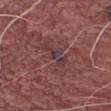notes = total-body-photography surveillance lesion; no biopsy | subject = male, about 80 years old | lesion diameter = ≈4 mm | image-analysis metrics = an area of roughly 5.5 mm², a shape eccentricity near 0.9, and two-axis asymmetry of about 0.5; a lesion color around L≈36 a*≈21 b*≈15 in CIELAB and a normalized lesion–skin contrast near 6.5; an automated nevus-likeness rating near 0 out of 100 and a detector confidence of about 95 out of 100 that the crop contains a lesion | anatomic site = the left thigh | tile lighting = white-light | image source = total-body-photography crop, ~15 mm field of view.